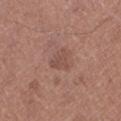| feature | finding |
|---|---|
| biopsy status | imaged on a skin check; not biopsied |
| image source | 15 mm crop, total-body photography |
| anatomic site | the left lower leg |
| patient | female, aged 48 to 52 |